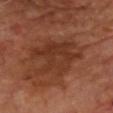No biopsy was performed on this lesion — it was imaged during a full skin examination and was not determined to be concerning.